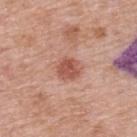Q: What kind of image is this?
A: ~15 mm crop, total-body skin-cancer survey
Q: Where on the body is the lesion?
A: the upper back
Q: What are the patient's age and sex?
A: female, approximately 60 years of age
Q: What did the biopsy show?
A: a compound melanocytic nevus